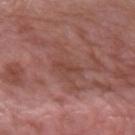Case summary:
• workup — catalogued during a skin exam; not biopsied
• patient — male, aged 38 to 42
• automated lesion analysis — a footprint of about 2 mm², an eccentricity of roughly 0.9, and a shape-asymmetry score of about 0.25 (0 = symmetric); about 6 CIELAB-L* units darker than the surrounding skin and a lesion-to-skin contrast of about 5.5 (normalized; higher = more distinct); a detector confidence of about 100 out of 100 that the crop contains a lesion
• site — the left forearm
• lesion size — ~2.5 mm (longest diameter)
• tile lighting — white-light illumination
• acquisition — 15 mm crop, total-body photography